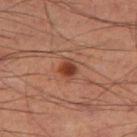The lesion was photographed on a routine skin check and not biopsied; there is no pathology result.
From the leg.
A lesion tile, about 15 mm wide, cut from a 3D total-body photograph.
The patient is a male about 60 years old.
The lesion's longest dimension is about 2 mm.
The lesion-visualizer software estimated a footprint of about 4 mm², an outline eccentricity of about 0.5 (0 = round, 1 = elongated), and a shape-asymmetry score of about 0.2 (0 = symmetric). The analysis additionally found an average lesion color of about L≈37 a*≈24 b*≈29 (CIELAB) and a normalized border contrast of about 9. The analysis additionally found a color-variation rating of about 3.5/10 and a peripheral color-asymmetry measure near 1. The software also gave a nevus-likeness score of about 100/100 and a detector confidence of about 100 out of 100 that the crop contains a lesion.